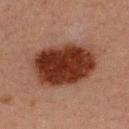{"biopsy_status": "not biopsied; imaged during a skin examination", "lighting": "cross-polarized", "image": {"source": "total-body photography crop", "field_of_view_mm": 15}, "lesion_size": {"long_diameter_mm_approx": 7.5}, "automated_metrics": {"area_mm2_approx": 32.0, "eccentricity": 0.7, "shape_asymmetry": 0.1, "cielab_L": 28, "cielab_a": 22, "cielab_b": 26, "vs_skin_darker_L": 16.0, "vs_skin_contrast_norm": 14.5, "border_irregularity_0_10": 1.5, "peripheral_color_asymmetry": 1.5, "lesion_detection_confidence_0_100": 100}, "patient": {"sex": "male", "age_approx": 30}, "site": "upper back"}